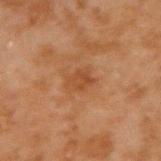Q: Was this lesion biopsied?
A: total-body-photography surveillance lesion; no biopsy
Q: Who is the patient?
A: male, aged around 45
Q: What kind of image is this?
A: total-body-photography crop, ~15 mm field of view
Q: Lesion size?
A: about 3 mm
Q: What is the anatomic site?
A: the right upper arm
Q: What lighting was used for the tile?
A: cross-polarized